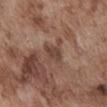| key | value |
|---|---|
| follow-up | imaged on a skin check; not biopsied |
| subject | male, approximately 75 years of age |
| location | the front of the torso |
| image | ~15 mm crop, total-body skin-cancer survey |
| automated metrics | a footprint of about 4.5 mm² and two-axis asymmetry of about 0.25; an automated nevus-likeness rating near 0 out of 100 and a detector confidence of about 100 out of 100 that the crop contains a lesion |
| tile lighting | white-light |